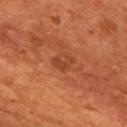Clinical impression:
The lesion was tiled from a total-body skin photograph and was not biopsied.
Acquisition and patient details:
Measured at roughly 2.5 mm in maximum diameter. This image is a 15 mm lesion crop taken from a total-body photograph. The lesion is located on the chest. The total-body-photography lesion software estimated a mean CIELAB color near L≈40 a*≈27 b*≈34 and a normalized lesion–skin contrast near 5.5. The analysis additionally found a lesion-detection confidence of about 100/100. The subject is a male aged 53–57.